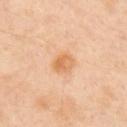workup: imaged on a skin check; not biopsied | site: the arm | tile lighting: cross-polarized illumination | image: ~15 mm tile from a whole-body skin photo | subject: male, in their 50s.